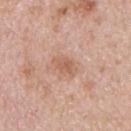| field | value |
|---|---|
| anatomic site | the upper back |
| image-analysis metrics | a lesion area of about 5 mm², an outline eccentricity of about 0.8 (0 = round, 1 = elongated), and a shape-asymmetry score of about 0.2 (0 = symmetric); a lesion color around L≈60 a*≈22 b*≈30 in CIELAB, a lesion–skin lightness drop of about 8, and a normalized border contrast of about 6 |
| patient | female, aged 43 to 47 |
| lighting | white-light illumination |
| image | ~15 mm crop, total-body skin-cancer survey |